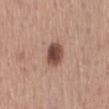notes: total-body-photography surveillance lesion; no biopsy | image: ~15 mm tile from a whole-body skin photo | location: the mid back | size: ≈3 mm | subject: male, roughly 65 years of age.